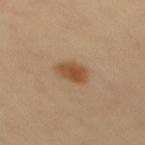Assessment: The lesion was tiled from a total-body skin photograph and was not biopsied. Clinical summary: A female subject aged around 50. The lesion-visualizer software estimated an average lesion color of about L≈48 a*≈19 b*≈35 (CIELAB), roughly 11 lightness units darker than nearby skin, and a normalized border contrast of about 9. The software also gave a classifier nevus-likeness of about 100/100. A close-up tile cropped from a whole-body skin photograph, about 15 mm across.